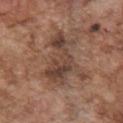Recorded during total-body skin imaging; not selected for excision or biopsy. A male patient, aged 73 to 77. The lesion is located on the chest. A lesion tile, about 15 mm wide, cut from a 3D total-body photograph.